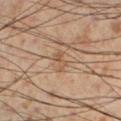Q: How was this image acquired?
A: ~15 mm tile from a whole-body skin photo
Q: What lighting was used for the tile?
A: cross-polarized
Q: What is the anatomic site?
A: the left lower leg
Q: Patient demographics?
A: male, in their mid- to late 50s